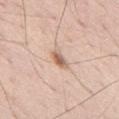| feature | finding |
|---|---|
| follow-up | imaged on a skin check; not biopsied |
| location | the mid back |
| subject | male, approximately 70 years of age |
| image | ~15 mm tile from a whole-body skin photo |
| diameter | ≈2.5 mm |
| tile lighting | white-light illumination |